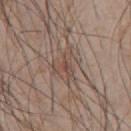Captured during whole-body skin photography for melanoma surveillance; the lesion was not biopsied. The lesion is on the chest. A male patient, aged 43 to 47. A roughly 15 mm field-of-view crop from a total-body skin photograph.